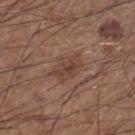biopsy status: imaged on a skin check; not biopsied | diameter: about 4 mm | subject: male, roughly 60 years of age | image: ~15 mm crop, total-body skin-cancer survey | illumination: white-light illumination | location: the right lower leg | automated lesion analysis: a lesion area of about 7.5 mm², an eccentricity of roughly 0.8, and a shape-asymmetry score of about 0.2 (0 = symmetric); a border-irregularity rating of about 2.5/10, internal color variation of about 3.5 on a 0–10 scale, and a peripheral color-asymmetry measure near 1.5; a classifier nevus-likeness of about 10/100 and lesion-presence confidence of about 100/100.